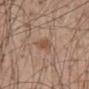<case>
  <biopsy_status>not biopsied; imaged during a skin examination</biopsy_status>
  <site>mid back</site>
  <automated_metrics>
    <area_mm2_approx>4.0</area_mm2_approx>
    <shape_asymmetry>0.3</shape_asymmetry>
    <cielab_L>51</cielab_L>
    <cielab_a>19</cielab_a>
    <cielab_b>30</cielab_b>
    <vs_skin_darker_L>7.0</vs_skin_darker_L>
    <vs_skin_contrast_norm>6.5</vs_skin_contrast_norm>
    <border_irregularity_0_10>3.5</border_irregularity_0_10>
    <color_variation_0_10>1.0</color_variation_0_10>
    <peripheral_color_asymmetry>0.5</peripheral_color_asymmetry>
  </automated_metrics>
  <lesion_size>
    <long_diameter_mm_approx>3.5</long_diameter_mm_approx>
  </lesion_size>
  <patient>
    <sex>male</sex>
    <age_approx>50</age_approx>
  </patient>
  <image>
    <source>total-body photography crop</source>
    <field_of_view_mm>15</field_of_view_mm>
  </image>
</case>